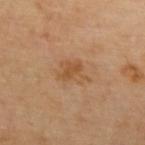| key | value |
|---|---|
| follow-up | catalogued during a skin exam; not biopsied |
| body site | the upper back |
| tile lighting | cross-polarized illumination |
| acquisition | ~15 mm crop, total-body skin-cancer survey |
| patient | female, in their mid-60s |
| TBP lesion metrics | an area of roughly 7 mm² and a shape eccentricity near 0.65; a lesion color around L≈52 a*≈19 b*≈36 in CIELAB and roughly 7 lightness units darker than nearby skin; a nevus-likeness score of about 0/100 |